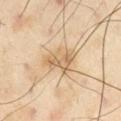No biopsy was performed on this lesion — it was imaged during a full skin examination and was not determined to be concerning. This is a cross-polarized tile. A 15 mm close-up extracted from a 3D total-body photography capture. The lesion-visualizer software estimated a lesion area of about 8.5 mm², an outline eccentricity of about 0.75 (0 = round, 1 = elongated), and a symmetry-axis asymmetry near 0.4. It also reported a border-irregularity rating of about 4.5/10. The software also gave a nevus-likeness score of about 0/100 and lesion-presence confidence of about 100/100. On the right thigh. The patient is a male aged around 55. Measured at roughly 4 mm in maximum diameter.